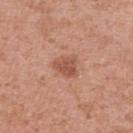Q: Was this lesion biopsied?
A: no biopsy performed (imaged during a skin exam)
Q: What kind of image is this?
A: 15 mm crop, total-body photography
Q: What is the lesion's diameter?
A: ≈2.5 mm
Q: What lighting was used for the tile?
A: white-light illumination
Q: What did automated image analysis measure?
A: an outline eccentricity of about 0.4 (0 = round, 1 = elongated) and a symmetry-axis asymmetry near 0.35; a lesion–skin lightness drop of about 10 and a lesion-to-skin contrast of about 7 (normalized; higher = more distinct)
Q: Where on the body is the lesion?
A: the right upper arm
Q: Patient demographics?
A: female, aged 28–32